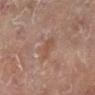The lesion was photographed on a routine skin check and not biopsied; there is no pathology result. From the left leg. This image is a 15 mm lesion crop taken from a total-body photograph. A female patient, about 80 years old.